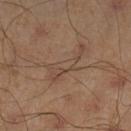Clinical impression:
The lesion was tiled from a total-body skin photograph and was not biopsied.
Context:
The lesion's longest dimension is about 5.5 mm. The lesion is on the left lower leg. A 15 mm crop from a total-body photograph taken for skin-cancer surveillance. The patient is a male approximately 45 years of age.